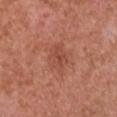| field | value |
|---|---|
| follow-up | imaged on a skin check; not biopsied |